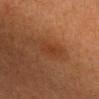biopsy status: catalogued during a skin exam; not biopsied
lesion diameter: ≈3 mm
imaging modality: ~15 mm crop, total-body skin-cancer survey
location: the head or neck
subject: female, approximately 40 years of age
automated metrics: a shape eccentricity near 0.8 and a shape-asymmetry score of about 0.25 (0 = symmetric); a mean CIELAB color near L≈33 a*≈21 b*≈30, a lesion–skin lightness drop of about 5, and a normalized border contrast of about 5; a border-irregularity rating of about 3/10 and radial color variation of about 0.5; a classifier nevus-likeness of about 0/100 and a lesion-detection confidence of about 100/100
lighting: cross-polarized